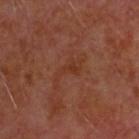Part of a total-body skin-imaging series; this lesion was reviewed on a skin check and was not flagged for biopsy.
About 5 mm across.
The patient is a male aged 58–62.
Located on the head or neck.
A 15 mm crop from a total-body photograph taken for skin-cancer surveillance.
Imaged with cross-polarized lighting.
An algorithmic analysis of the crop reported a lesion area of about 5.5 mm², a shape eccentricity near 0.9, and two-axis asymmetry of about 0.6. The analysis additionally found a mean CIELAB color near L≈34 a*≈24 b*≈29, a lesion–skin lightness drop of about 4, and a normalized border contrast of about 5.